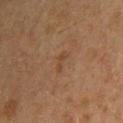Case summary:
- image · ~15 mm tile from a whole-body skin photo
- TBP lesion metrics · an area of roughly 2 mm² and two-axis asymmetry of about 0.5
- tile lighting · cross-polarized
- patient · male, approximately 60 years of age